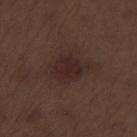The tile uses white-light illumination.
Measured at roughly 4 mm in maximum diameter.
Automated image analysis of the tile measured a classifier nevus-likeness of about 50/100.
A lesion tile, about 15 mm wide, cut from a 3D total-body photograph.
From the left thigh.
The subject is a male roughly 70 years of age.